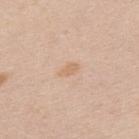{
  "biopsy_status": "not biopsied; imaged during a skin examination",
  "site": "upper back",
  "image": {
    "source": "total-body photography crop",
    "field_of_view_mm": 15
  },
  "automated_metrics": {
    "border_irregularity_0_10": 3.0,
    "color_variation_0_10": 1.0
  },
  "lighting": "white-light",
  "patient": {
    "sex": "male",
    "age_approx": 35
  },
  "lesion_size": {
    "long_diameter_mm_approx": 2.5
  }
}